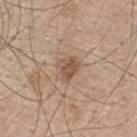No biopsy was performed on this lesion — it was imaged during a full skin examination and was not determined to be concerning.
The tile uses white-light illumination.
From the back.
The subject is a male aged approximately 50.
A close-up tile cropped from a whole-body skin photograph, about 15 mm across.
An algorithmic analysis of the crop reported a lesion area of about 5 mm², an eccentricity of roughly 0.55, and two-axis asymmetry of about 0.3. It also reported an average lesion color of about L≈53 a*≈18 b*≈30 (CIELAB), roughly 10 lightness units darker than nearby skin, and a lesion-to-skin contrast of about 7.5 (normalized; higher = more distinct). And it measured a border-irregularity index near 2.5/10, a color-variation rating of about 2.5/10, and peripheral color asymmetry of about 1. It also reported lesion-presence confidence of about 100/100.
Longest diameter approximately 2.5 mm.